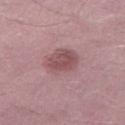follow-up = no biopsy performed (imaged during a skin exam) | location = the left thigh | automated lesion analysis = an area of roughly 15 mm², a shape eccentricity near 0.35, and a symmetry-axis asymmetry near 0.15; a border-irregularity rating of about 1.5/10, a color-variation rating of about 4/10, and a peripheral color-asymmetry measure near 1.5 | acquisition = ~15 mm tile from a whole-body skin photo | patient = male, in their 30s | lesion diameter = ≈4.5 mm.